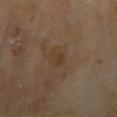{
  "biopsy_status": "not biopsied; imaged during a skin examination",
  "lesion_size": {
    "long_diameter_mm_approx": 2.5
  },
  "patient": {
    "sex": "male",
    "age_approx": 65
  },
  "lighting": "cross-polarized",
  "site": "right upper arm",
  "image": {
    "source": "total-body photography crop",
    "field_of_view_mm": 15
  }
}